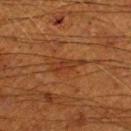Clinical impression:
The lesion was tiled from a total-body skin photograph and was not biopsied.
Clinical summary:
A lesion tile, about 15 mm wide, cut from a 3D total-body photograph. The lesion's longest dimension is about 4 mm. The total-body-photography lesion software estimated a lesion area of about 4.5 mm², an eccentricity of roughly 0.95, and a shape-asymmetry score of about 0.3 (0 = symmetric). It also reported border irregularity of about 4 on a 0–10 scale, a color-variation rating of about 1.5/10, and peripheral color asymmetry of about 0. And it measured an automated nevus-likeness rating near 0 out of 100. A male patient, approximately 60 years of age. The lesion is on the leg.